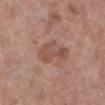The lesion was photographed on a routine skin check and not biopsied; there is no pathology result. A female subject, roughly 50 years of age. The lesion is on the right lower leg. Longest diameter approximately 4.5 mm. Imaged with white-light lighting. Cropped from a whole-body photographic skin survey; the tile spans about 15 mm.